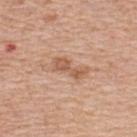{
  "image": {
    "source": "total-body photography crop",
    "field_of_view_mm": 15
  },
  "patient": {
    "sex": "female",
    "age_approx": 50
  },
  "site": "back",
  "lesion_size": {
    "long_diameter_mm_approx": 4.0
  }
}